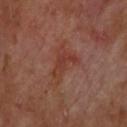follow-up — catalogued during a skin exam; not biopsied | image source — 15 mm crop, total-body photography | illumination — cross-polarized illumination | body site — the upper back | diameter — ≈5 mm | subject — male, about 70 years old | image-analysis metrics — an area of roughly 8 mm², an outline eccentricity of about 0.8 (0 = round, 1 = elongated), and a symmetry-axis asymmetry near 0.55; a nevus-likeness score of about 0/100 and a lesion-detection confidence of about 100/100.